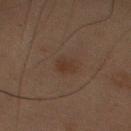No biopsy was performed on this lesion — it was imaged during a full skin examination and was not determined to be concerning.
This is a cross-polarized tile.
A 15 mm close-up tile from a total-body photography series done for melanoma screening.
The recorded lesion diameter is about 2.5 mm.
Automated tile analysis of the lesion measured a lesion area of about 3 mm², an outline eccentricity of about 0.75 (0 = round, 1 = elongated), and two-axis asymmetry of about 0.3. The software also gave a border-irregularity rating of about 3.5/10 and internal color variation of about 1 on a 0–10 scale.
The lesion is on the right upper arm.
A male patient, about 70 years old.